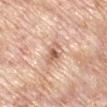This lesion was catalogued during total-body skin photography and was not selected for biopsy. Located on the left upper arm. A male patient, aged 68 to 72. Longest diameter approximately 3.5 mm. A lesion tile, about 15 mm wide, cut from a 3D total-body photograph.